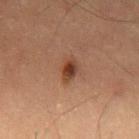| key | value |
|---|---|
| follow-up | catalogued during a skin exam; not biopsied |
| image | 15 mm crop, total-body photography |
| diameter | ≈3 mm |
| illumination | cross-polarized illumination |
| patient | male, aged around 60 |
| TBP lesion metrics | an area of roughly 5 mm², an eccentricity of roughly 0.8, and two-axis asymmetry of about 0.25; a mean CIELAB color near L≈36 a*≈20 b*≈27 and a normalized lesion–skin contrast near 9; a border-irregularity index near 2.5/10, internal color variation of about 5 on a 0–10 scale, and a peripheral color-asymmetry measure near 1.5 |